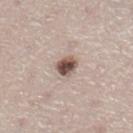tile lighting = white-light illumination | location = the leg | subject = male, aged around 60 | imaging modality = ~15 mm crop, total-body skin-cancer survey | diameter = about 2.5 mm.